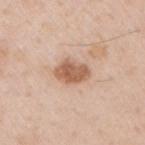Captured during whole-body skin photography for melanoma surveillance; the lesion was not biopsied. From the right upper arm. A 15 mm crop from a total-body photograph taken for skin-cancer surveillance. The tile uses white-light illumination. A male subject, in their 50s.